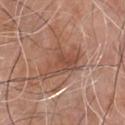| key | value |
|---|---|
| follow-up | no biopsy performed (imaged during a skin exam) |
| image-analysis metrics | a lesion area of about 13 mm², a shape eccentricity near 0.8, and two-axis asymmetry of about 0.45; a lesion color around L≈50 a*≈21 b*≈29 in CIELAB and a normalized border contrast of about 6.5; a border-irregularity index near 5.5/10, a within-lesion color-variation index near 4.5/10, and radial color variation of about 1.5 |
| lesion size | about 5.5 mm |
| imaging modality | ~15 mm crop, total-body skin-cancer survey |
| patient | male, aged around 80 |
| location | the chest |